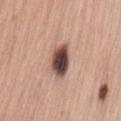Case summary:
• workup · no biopsy performed (imaged during a skin exam)